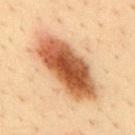Clinical impression: The lesion was tiled from a total-body skin photograph and was not biopsied. Background: A male subject approximately 35 years of age. On the mid back. A 15 mm crop from a total-body photograph taken for skin-cancer surveillance.